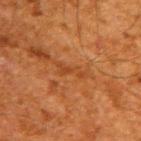patient = male, about 60 years old
body site = the upper back
lesion diameter = ~3.5 mm (longest diameter)
image source = ~15 mm crop, total-body skin-cancer survey
lighting = cross-polarized illumination